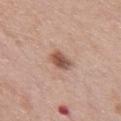The lesion was photographed on a routine skin check and not biopsied; there is no pathology result. On the chest. A female subject in their mid-60s. A 15 mm crop from a total-body photograph taken for skin-cancer surveillance.